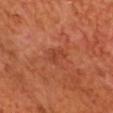The lesion was tiled from a total-body skin photograph and was not biopsied. The recorded lesion diameter is about 3 mm. A male patient, in their mid- to late 60s. Cropped from a whole-body photographic skin survey; the tile spans about 15 mm. An algorithmic analysis of the crop reported an average lesion color of about L≈42 a*≈30 b*≈34 (CIELAB). And it measured border irregularity of about 4.5 on a 0–10 scale, internal color variation of about 1 on a 0–10 scale, and peripheral color asymmetry of about 0.5. Captured under cross-polarized illumination.